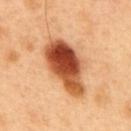The lesion was photographed on a routine skin check and not biopsied; there is no pathology result.
The total-body-photography lesion software estimated a lesion area of about 21 mm², a shape eccentricity near 0.85, and a symmetry-axis asymmetry near 0.25. And it measured a mean CIELAB color near L≈51 a*≈30 b*≈39, a lesion–skin lightness drop of about 22, and a normalized border contrast of about 14. It also reported border irregularity of about 3 on a 0–10 scale, a color-variation rating of about 10/10, and radial color variation of about 3.
A 15 mm crop from a total-body photograph taken for skin-cancer surveillance.
Captured under cross-polarized illumination.
The patient is a male aged around 50.
The lesion is located on the upper back.
The recorded lesion diameter is about 7.5 mm.